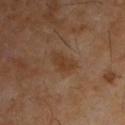Imaged during a routine full-body skin examination; the lesion was not biopsied and no histopathology is available. The patient is a male in their mid- to late 50s. A roughly 15 mm field-of-view crop from a total-body skin photograph. The lesion is on the upper back. An algorithmic analysis of the crop reported a mean CIELAB color near L≈35 a*≈17 b*≈29, roughly 6 lightness units darker than nearby skin, and a lesion-to-skin contrast of about 6.5 (normalized; higher = more distinct). And it measured a border-irregularity rating of about 3.5/10, a color-variation rating of about 2/10, and peripheral color asymmetry of about 0.5.